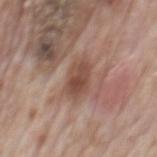{"biopsy_status": "not biopsied; imaged during a skin examination", "lighting": "white-light", "patient": {"sex": "male", "age_approx": 70}, "site": "mid back", "image": {"source": "total-body photography crop", "field_of_view_mm": 15}}